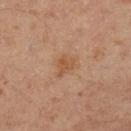| field | value |
|---|---|
| follow-up | imaged on a skin check; not biopsied |